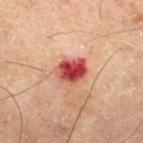The lesion was tiled from a total-body skin photograph and was not biopsied.
Automated tile analysis of the lesion measured a nevus-likeness score of about 0/100 and lesion-presence confidence of about 100/100.
A male subject, aged approximately 65.
A 15 mm close-up extracted from a 3D total-body photography capture.
Located on the right thigh.
Approximately 3.5 mm at its widest.